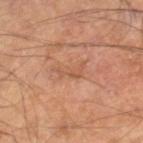  biopsy_status: not biopsied; imaged during a skin examination
  site: leg
  lighting: cross-polarized
  patient:
    sex: male
    age_approx: 65
  automated_metrics:
    area_mm2_approx: 3.5
    cielab_L: 53
    cielab_a: 23
    cielab_b: 32
    vs_skin_darker_L: 7.0
    vs_skin_contrast_norm: 5.0
    nevus_likeness_0_100: 0
    lesion_detection_confidence_0_100: 95
  lesion_size:
    long_diameter_mm_approx: 3.5
  image:
    source: total-body photography crop
    field_of_view_mm: 15